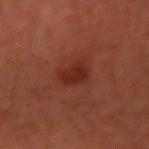Part of a total-body skin-imaging series; this lesion was reviewed on a skin check and was not flagged for biopsy.
The tile uses cross-polarized illumination.
Located on the right upper arm.
Automated image analysis of the tile measured an area of roughly 6.5 mm², an outline eccentricity of about 0.7 (0 = round, 1 = elongated), and a shape-asymmetry score of about 0.3 (0 = symmetric).
Approximately 3.5 mm at its widest.
A male subject, roughly 60 years of age.
A lesion tile, about 15 mm wide, cut from a 3D total-body photograph.